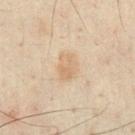  biopsy_status: not biopsied; imaged during a skin examination
  patient:
    sex: male
    age_approx: 50
  image:
    source: total-body photography crop
    field_of_view_mm: 15
  lighting: cross-polarized
  lesion_size:
    long_diameter_mm_approx: 3.5
  site: chest
  automated_metrics:
    area_mm2_approx: 7.5
    eccentricity: 0.7
    shape_asymmetry: 0.2
    cielab_L: 61
    cielab_a: 13
    cielab_b: 31
    vs_skin_contrast_norm: 5.0
    border_irregularity_0_10: 2.0
    color_variation_0_10: 2.5
    peripheral_color_asymmetry: 1.0
    nevus_likeness_0_100: 10
    lesion_detection_confidence_0_100: 100